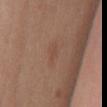Clinical impression: No biopsy was performed on this lesion — it was imaged during a full skin examination and was not determined to be concerning. Clinical summary: This is a white-light tile. The subject is a female aged approximately 55. A roughly 15 mm field-of-view crop from a total-body skin photograph. The lesion is on the abdomen. The recorded lesion diameter is about 2.5 mm.